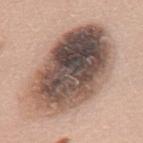{"biopsy_status": "not biopsied; imaged during a skin examination", "site": "mid back", "image": {"source": "total-body photography crop", "field_of_view_mm": 15}, "patient": {"sex": "female", "age_approx": 60}}